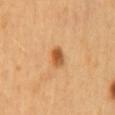follow-up: no biopsy performed (imaged during a skin exam) | illumination: cross-polarized illumination | lesion size: ≈2.5 mm | subject: female, aged 58 to 62 | body site: the back | automated metrics: an average lesion color of about L≈58 a*≈26 b*≈44 (CIELAB), a lesion–skin lightness drop of about 14, and a lesion-to-skin contrast of about 9 (normalized; higher = more distinct); a nevus-likeness score of about 95/100 | image: 15 mm crop, total-body photography.